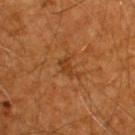Notes:
* follow-up · catalogued during a skin exam; not biopsied
* image source · 15 mm crop, total-body photography
* site · the back
* patient · male, roughly 60 years of age